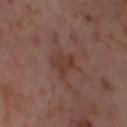This lesion was catalogued during total-body skin photography and was not selected for biopsy.
A roughly 15 mm field-of-view crop from a total-body skin photograph.
On the left thigh.
A female patient approximately 55 years of age.
This is a cross-polarized tile.
About 3.5 mm across.
Automated tile analysis of the lesion measured a border-irregularity rating of about 4/10, a color-variation rating of about 2.5/10, and a peripheral color-asymmetry measure near 1. The software also gave a nevus-likeness score of about 0/100 and a lesion-detection confidence of about 100/100.